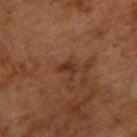Clinical impression:
Captured during whole-body skin photography for melanoma surveillance; the lesion was not biopsied.